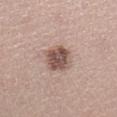{
  "biopsy_status": "not biopsied; imaged during a skin examination",
  "patient": {
    "sex": "female",
    "age_approx": 35
  },
  "lighting": "white-light",
  "automated_metrics": {
    "area_mm2_approx": 9.0,
    "eccentricity": 0.65,
    "nevus_likeness_0_100": 40,
    "lesion_detection_confidence_0_100": 100
  },
  "image": {
    "source": "total-body photography crop",
    "field_of_view_mm": 15
  },
  "site": "left lower leg"
}